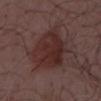biopsy_status: not biopsied; imaged during a skin examination
patient:
  sex: male
  age_approx: 55
site: chest
image:
  source: total-body photography crop
  field_of_view_mm: 15